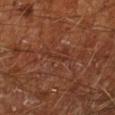<record>
<biopsy_status>not biopsied; imaged during a skin examination</biopsy_status>
<image>
  <source>total-body photography crop</source>
  <field_of_view_mm>15</field_of_view_mm>
</image>
<site>left lower leg</site>
<patient>
  <sex>male</sex>
  <age_approx>65</age_approx>
</patient>
<lesion_size>
  <long_diameter_mm_approx>2.5</long_diameter_mm_approx>
</lesion_size>
<lighting>cross-polarized</lighting>
</record>